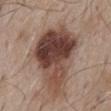biopsy status — catalogued during a skin exam; not biopsied | size — ~9 mm (longest diameter) | image-analysis metrics — an area of roughly 33 mm², an outline eccentricity of about 0.8 (0 = round, 1 = elongated), and two-axis asymmetry of about 0.4; a lesion color around L≈42 a*≈19 b*≈24 in CIELAB and a lesion–skin lightness drop of about 17; internal color variation of about 9.5 on a 0–10 scale and a peripheral color-asymmetry measure near 3.5; an automated nevus-likeness rating near 5 out of 100 and lesion-presence confidence of about 100/100 | body site — the back | acquisition — 15 mm crop, total-body photography | patient — male, aged 53 to 57.